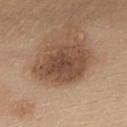Recorded during total-body skin imaging; not selected for excision or biopsy.
The lesion is located on the front of the torso.
A female subject aged around 65.
Imaged with white-light lighting.
The total-body-photography lesion software estimated roughly 13 lightness units darker than nearby skin and a lesion-to-skin contrast of about 9 (normalized; higher = more distinct). The analysis additionally found a border-irregularity rating of about 2.5/10, internal color variation of about 3.5 on a 0–10 scale, and radial color variation of about 1. And it measured a nevus-likeness score of about 15/100 and a detector confidence of about 100 out of 100 that the crop contains a lesion.
A close-up tile cropped from a whole-body skin photograph, about 15 mm across.
The lesion's longest dimension is about 7 mm.